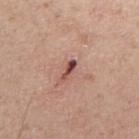workup: total-body-photography surveillance lesion; no biopsy | subject: female, approximately 40 years of age | tile lighting: white-light | site: the right upper arm | image: 15 mm crop, total-body photography.